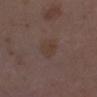Part of a total-body skin-imaging series; this lesion was reviewed on a skin check and was not flagged for biopsy.
A female patient, about 35 years old.
The total-body-photography lesion software estimated an area of roughly 6.5 mm², an outline eccentricity of about 0.55 (0 = round, 1 = elongated), and a shape-asymmetry score of about 0.2 (0 = symmetric). The software also gave a mean CIELAB color near L≈37 a*≈15 b*≈21 and roughly 4 lightness units darker than nearby skin. The analysis additionally found a classifier nevus-likeness of about 0/100 and lesion-presence confidence of about 100/100.
The tile uses white-light illumination.
Located on the leg.
A region of skin cropped from a whole-body photographic capture, roughly 15 mm wide.